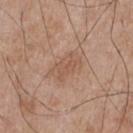Clinical impression: This lesion was catalogued during total-body skin photography and was not selected for biopsy. Clinical summary: A 15 mm close-up tile from a total-body photography series done for melanoma screening. Captured under white-light illumination. The recorded lesion diameter is about 4 mm. Automated tile analysis of the lesion measured a lesion color around L≈55 a*≈19 b*≈29 in CIELAB and a normalized lesion–skin contrast near 5. And it measured a border-irregularity index near 3/10 and a peripheral color-asymmetry measure near 1. And it measured a nevus-likeness score of about 0/100 and a lesion-detection confidence of about 100/100. Located on the chest. The patient is a male in their mid- to late 60s.